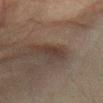Findings:
• acquisition — ~15 mm tile from a whole-body skin photo
• subject — female, aged approximately 45
• body site — the chest
• lesion size — about 4 mm